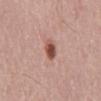Recorded during total-body skin imaging; not selected for excision or biopsy. The tile uses white-light illumination. A male subject aged 58 to 62. This image is a 15 mm lesion crop taken from a total-body photograph. On the mid back.